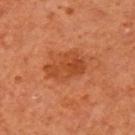biopsy status = imaged on a skin check; not biopsied